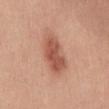biopsy status=total-body-photography surveillance lesion; no biopsy | patient=female, approximately 45 years of age | imaging modality=15 mm crop, total-body photography | anatomic site=the chest.